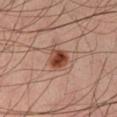The lesion was photographed on a routine skin check and not biopsied; there is no pathology result. A male subject in their mid- to late 40s. Located on the left thigh. A lesion tile, about 15 mm wide, cut from a 3D total-body photograph. Automated tile analysis of the lesion measured a lesion color around L≈42 a*≈22 b*≈28 in CIELAB and a lesion–skin lightness drop of about 13. The analysis additionally found a border-irregularity rating of about 2.5/10. The analysis additionally found a nevus-likeness score of about 100/100. The tile uses cross-polarized illumination.